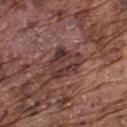Notes:
- workup: catalogued during a skin exam; not biopsied